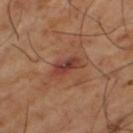workup: total-body-photography surveillance lesion; no biopsy | acquisition: ~15 mm tile from a whole-body skin photo | body site: the left thigh | subject: male, aged around 65 | lighting: cross-polarized | lesion diameter: ~3.5 mm (longest diameter).